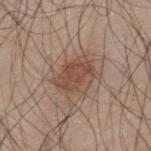Q: Is there a histopathology result?
A: imaged on a skin check; not biopsied
Q: What is the anatomic site?
A: the upper back
Q: What lighting was used for the tile?
A: white-light illumination
Q: What is the imaging modality?
A: total-body-photography crop, ~15 mm field of view
Q: Patient demographics?
A: male, roughly 45 years of age
Q: Lesion size?
A: ~4.5 mm (longest diameter)